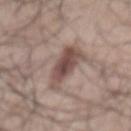• biopsy status — imaged on a skin check; not biopsied
• imaging modality — ~15 mm crop, total-body skin-cancer survey
• subject — male, aged 48 to 52
• size — about 6.5 mm
• illumination — white-light illumination
• anatomic site — the back